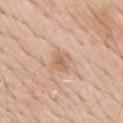Clinical impression: Recorded during total-body skin imaging; not selected for excision or biopsy. Clinical summary: Imaged with white-light lighting. A male subject in their mid- to late 60s. Measured at roughly 2.5 mm in maximum diameter. The total-body-photography lesion software estimated peripheral color asymmetry of about 1. It also reported a classifier nevus-likeness of about 0/100 and lesion-presence confidence of about 100/100. A roughly 15 mm field-of-view crop from a total-body skin photograph. On the chest.